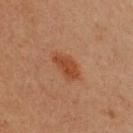notes=total-body-photography surveillance lesion; no biopsy | lesion diameter=≈4 mm | lighting=cross-polarized | imaging modality=~15 mm crop, total-body skin-cancer survey | patient=female, aged 48 to 52 | automated lesion analysis=a lesion color around L≈46 a*≈27 b*≈36 in CIELAB, a lesion–skin lightness drop of about 9, and a normalized lesion–skin contrast near 8.